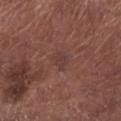This lesion was catalogued during total-body skin photography and was not selected for biopsy. Imaged with white-light lighting. About 2.5 mm across. A 15 mm close-up tile from a total-body photography series done for melanoma screening. Located on the leg. A male patient roughly 55 years of age. An algorithmic analysis of the crop reported an area of roughly 4 mm², a shape eccentricity near 0.7, and a shape-asymmetry score of about 0.3 (0 = symmetric). It also reported about 5 CIELAB-L* units darker than the surrounding skin. The analysis additionally found a color-variation rating of about 1/10.